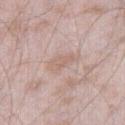patient = male, about 45 years old
tile lighting = white-light illumination
location = the right thigh
image = ~15 mm crop, total-body skin-cancer survey
size = about 4 mm